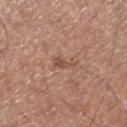Assessment: This lesion was catalogued during total-body skin photography and was not selected for biopsy. Image and clinical context: A 15 mm close-up tile from a total-body photography series done for melanoma screening. The subject is a male aged 63–67. The recorded lesion diameter is about 3 mm. The lesion-visualizer software estimated a border-irregularity index near 5/10, a within-lesion color-variation index near 0.5/10, and a peripheral color-asymmetry measure near 0. The analysis additionally found a nevus-likeness score of about 0/100. This is a white-light tile. The lesion is on the right lower leg.